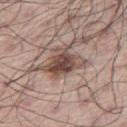Q: Is there a histopathology result?
A: imaged on a skin check; not biopsied
Q: What are the patient's age and sex?
A: male, aged 68 to 72
Q: What is the imaging modality?
A: ~15 mm tile from a whole-body skin photo
Q: Automated lesion metrics?
A: an area of roughly 10 mm², an outline eccentricity of about 0.85 (0 = round, 1 = elongated), and a symmetry-axis asymmetry near 0.35; an average lesion color of about L≈47 a*≈17 b*≈23 (CIELAB) and a normalized lesion–skin contrast near 10; a border-irregularity index near 4/10 and a within-lesion color-variation index near 6.5/10; a nevus-likeness score of about 95/100
Q: Where on the body is the lesion?
A: the left leg
Q: How was the tile lit?
A: white-light illumination